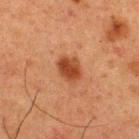No biopsy was performed on this lesion — it was imaged during a full skin examination and was not determined to be concerning. Captured under cross-polarized illumination. On the upper back. A male subject aged around 60. Cropped from a whole-body photographic skin survey; the tile spans about 15 mm. An algorithmic analysis of the crop reported an area of roughly 6 mm² and a shape-asymmetry score of about 0.15 (0 = symmetric). The software also gave a lesion color around L≈35 a*≈24 b*≈31 in CIELAB, roughly 11 lightness units darker than nearby skin, and a normalized border contrast of about 9.5. The software also gave border irregularity of about 1.5 on a 0–10 scale, a color-variation rating of about 3/10, and peripheral color asymmetry of about 1. It also reported a classifier nevus-likeness of about 100/100 and lesion-presence confidence of about 100/100. About 3 mm across.